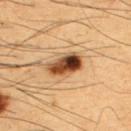biopsy status: no biopsy performed (imaged during a skin exam); patient: male, approximately 50 years of age; acquisition: 15 mm crop, total-body photography; lighting: cross-polarized; anatomic site: the back; diameter: ~4 mm (longest diameter).